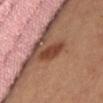{
  "biopsy_status": "not biopsied; imaged during a skin examination",
  "patient": {
    "sex": "female"
  },
  "site": "abdomen",
  "image": {
    "source": "total-body photography crop",
    "field_of_view_mm": 15
  }
}